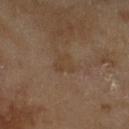Impression:
This lesion was catalogued during total-body skin photography and was not selected for biopsy.
Clinical summary:
A female patient, aged 58 to 62. On the left lower leg. A 15 mm close-up tile from a total-body photography series done for melanoma screening.